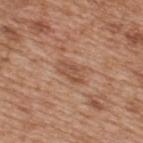Imaged during a routine full-body skin examination; the lesion was not biopsied and no histopathology is available.
Cropped from a whole-body photographic skin survey; the tile spans about 15 mm.
The lesion is on the back.
A male subject, in their mid- to late 60s.
Captured under white-light illumination.
Automated tile analysis of the lesion measured a within-lesion color-variation index near 3/10 and peripheral color asymmetry of about 1.